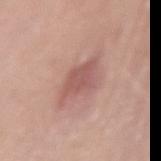This lesion was catalogued during total-body skin photography and was not selected for biopsy.
Cropped from a whole-body photographic skin survey; the tile spans about 15 mm.
On the lower back.
A female patient, in their 40s.
The lesion's longest dimension is about 5 mm.
The total-body-photography lesion software estimated an area of roughly 9.5 mm², an outline eccentricity of about 0.8 (0 = round, 1 = elongated), and a symmetry-axis asymmetry near 0.25. It also reported a border-irregularity rating of about 3.5/10 and radial color variation of about 0.5. The analysis additionally found an automated nevus-likeness rating near 15 out of 100.
Captured under white-light illumination.